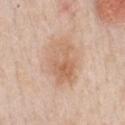Part of a total-body skin-imaging series; this lesion was reviewed on a skin check and was not flagged for biopsy.
Imaged with white-light lighting.
The lesion is located on the chest.
The subject is a male aged 58 to 62.
A 15 mm close-up extracted from a 3D total-body photography capture.